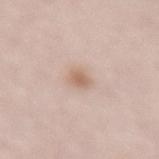The lesion was tiled from a total-body skin photograph and was not biopsied. Imaged with white-light lighting. From the lower back. A 15 mm close-up extracted from a 3D total-body photography capture. A female subject, approximately 50 years of age. The lesion's longest dimension is about 2.5 mm.